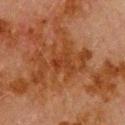Findings:
* notes — total-body-photography surveillance lesion; no biopsy
* automated lesion analysis — an area of roughly 4.5 mm² and a shape-asymmetry score of about 0.35 (0 = symmetric); an average lesion color of about L≈30 a*≈23 b*≈31 (CIELAB) and a lesion-to-skin contrast of about 5.5 (normalized; higher = more distinct); border irregularity of about 4.5 on a 0–10 scale and a peripheral color-asymmetry measure near 0.5
* patient — male, aged approximately 80
* image source — 15 mm crop, total-body photography
* site — the upper back
* lesion size — about 3 mm